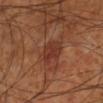The lesion was photographed on a routine skin check and not biopsied; there is no pathology result. The subject is a male aged around 70. On the leg. Cropped from a whole-body photographic skin survey; the tile spans about 15 mm. This is a cross-polarized tile. The lesion's longest dimension is about 3 mm.